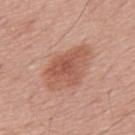The lesion was tiled from a total-body skin photograph and was not biopsied. Imaged with white-light lighting. A 15 mm close-up tile from a total-body photography series done for melanoma screening. The recorded lesion diameter is about 6 mm. The subject is a male aged 53–57.